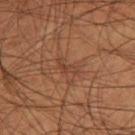Captured during whole-body skin photography for melanoma surveillance; the lesion was not biopsied.
On the right thigh.
Cropped from a total-body skin-imaging series; the visible field is about 15 mm.
A male patient, in their mid- to late 50s.